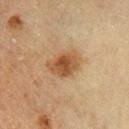<tbp_lesion>
  <site>left lower leg</site>
  <patient>
    <sex>male</sex>
    <age_approx>65</age_approx>
  </patient>
  <image>
    <source>total-body photography crop</source>
    <field_of_view_mm>15</field_of_view_mm>
  </image>
  <lesion_size>
    <long_diameter_mm_approx>4.0</long_diameter_mm_approx>
  </lesion_size>
</tbp_lesion>